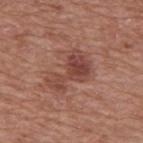workup: no biopsy performed (imaged during a skin exam) | size: ~5 mm (longest diameter) | TBP lesion metrics: a border-irregularity index near 6.5/10, internal color variation of about 4.5 on a 0–10 scale, and radial color variation of about 1; a nevus-likeness score of about 50/100 and a lesion-detection confidence of about 100/100 | patient: male, aged 73–77 | image: ~15 mm tile from a whole-body skin photo | illumination: white-light | body site: the upper back.